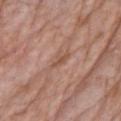{"biopsy_status": "not biopsied; imaged during a skin examination", "site": "chest", "lesion_size": {"long_diameter_mm_approx": 3.0}, "image": {"source": "total-body photography crop", "field_of_view_mm": 15}, "patient": {"sex": "female", "age_approx": 70}}